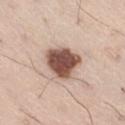Captured during whole-body skin photography for melanoma surveillance; the lesion was not biopsied. Automated tile analysis of the lesion measured a lesion color around L≈52 a*≈20 b*≈25 in CIELAB, about 21 CIELAB-L* units darker than the surrounding skin, and a normalized border contrast of about 13.5. The analysis additionally found a detector confidence of about 100 out of 100 that the crop contains a lesion. This image is a 15 mm lesion crop taken from a total-body photograph. The lesion is on the right thigh. The patient is a male aged around 55. The tile uses white-light illumination. About 4 mm across.